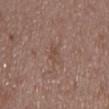Q: Is there a histopathology result?
A: catalogued during a skin exam; not biopsied
Q: Patient demographics?
A: male, roughly 50 years of age
Q: Lesion location?
A: the mid back
Q: How was this image acquired?
A: 15 mm crop, total-body photography
Q: How large is the lesion?
A: about 3 mm
Q: How was the tile lit?
A: white-light
Q: Automated lesion metrics?
A: a mean CIELAB color near L≈48 a*≈17 b*≈25 and a lesion-to-skin contrast of about 4.5 (normalized; higher = more distinct); a classifier nevus-likeness of about 0/100 and a detector confidence of about 90 out of 100 that the crop contains a lesion